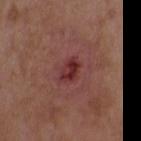notes = total-body-photography surveillance lesion; no biopsy | location = the upper back | subject = female, about 35 years old | imaging modality = ~15 mm crop, total-body skin-cancer survey.